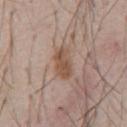{"biopsy_status": "not biopsied; imaged during a skin examination", "lighting": "white-light", "lesion_size": {"long_diameter_mm_approx": 4.0}, "image": {"source": "total-body photography crop", "field_of_view_mm": 15}, "patient": {"sex": "male", "age_approx": 55}}